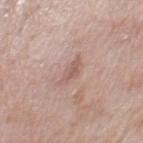Q: What are the patient's age and sex?
A: male, aged 68 to 72
Q: What did automated image analysis measure?
A: a border-irregularity rating of about 4/10, internal color variation of about 1.5 on a 0–10 scale, and a peripheral color-asymmetry measure near 0.5
Q: What is the anatomic site?
A: the chest
Q: What is the lesion's diameter?
A: about 3.5 mm
Q: What kind of image is this?
A: ~15 mm tile from a whole-body skin photo
Q: Illumination type?
A: white-light illumination